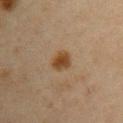Q: Was a biopsy performed?
A: imaged on a skin check; not biopsied
Q: How was this image acquired?
A: total-body-photography crop, ~15 mm field of view
Q: Lesion location?
A: the right upper arm
Q: Lesion size?
A: ~2.5 mm (longest diameter)
Q: Who is the patient?
A: female, aged around 45